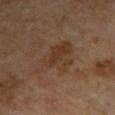Q: Was this lesion biopsied?
A: no biopsy performed (imaged during a skin exam)
Q: Lesion location?
A: the arm
Q: What did automated image analysis measure?
A: a classifier nevus-likeness of about 0/100 and a lesion-detection confidence of about 100/100
Q: Lesion size?
A: about 5 mm
Q: How was the tile lit?
A: cross-polarized illumination
Q: What kind of image is this?
A: 15 mm crop, total-body photography
Q: Who is the patient?
A: male, aged around 65